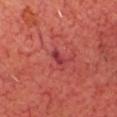{"biopsy_status": "not biopsied; imaged during a skin examination", "site": "head or neck", "image": {"source": "total-body photography crop", "field_of_view_mm": 15}, "lighting": "cross-polarized", "patient": {"sex": "male", "age_approx": 70}, "lesion_size": {"long_diameter_mm_approx": 2.5}, "automated_metrics": {"area_mm2_approx": 3.0, "eccentricity": 0.85, "shape_asymmetry": 0.3}}